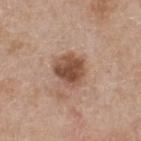Acquisition and patient details:
A lesion tile, about 15 mm wide, cut from a 3D total-body photograph. A male patient, about 55 years old. Captured under white-light illumination. About 4 mm across. The total-body-photography lesion software estimated a footprint of about 11 mm² and an eccentricity of roughly 0.45. The software also gave a lesion color around L≈50 a*≈20 b*≈29 in CIELAB, a lesion–skin lightness drop of about 13, and a normalized border contrast of about 9.5.